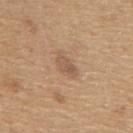No biopsy was performed on this lesion — it was imaged during a full skin examination and was not determined to be concerning. This image is a 15 mm lesion crop taken from a total-body photograph. The subject is a male in their mid- to late 60s. The lesion is on the mid back.